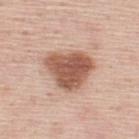biopsy status: no biopsy performed (imaged during a skin exam)
body site: the upper back
patient: male, aged 43 to 47
image: 15 mm crop, total-body photography